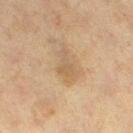Imaged during a routine full-body skin examination; the lesion was not biopsied and no histopathology is available.